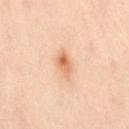Part of a total-body skin-imaging series; this lesion was reviewed on a skin check and was not flagged for biopsy. Approximately 3 mm at its widest. On the lower back. A close-up tile cropped from a whole-body skin photograph, about 15 mm across. A female patient, about 40 years old. Imaged with cross-polarized lighting.